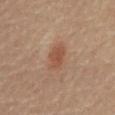– anatomic site — the mid back
– tile lighting — cross-polarized
– image-analysis metrics — an automated nevus-likeness rating near 95 out of 100 and a lesion-detection confidence of about 100/100
– image — 15 mm crop, total-body photography
– diameter — ~4 mm (longest diameter)
– subject — male, roughly 85 years of age or older